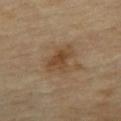notes: catalogued during a skin exam; not biopsied
image source: 15 mm crop, total-body photography
location: the mid back
subject: male, aged approximately 70
tile lighting: cross-polarized